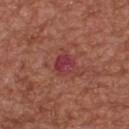patient — male, aged 63–67
site — the upper back
image-analysis metrics — a lesion color around L≈39 a*≈32 b*≈21 in CIELAB, roughly 8 lightness units darker than nearby skin, and a lesion-to-skin contrast of about 8.5 (normalized; higher = more distinct); border irregularity of about 2 on a 0–10 scale, a within-lesion color-variation index near 4/10, and peripheral color asymmetry of about 1.5
tile lighting — white-light illumination
size — ≈2.5 mm
image source — total-body-photography crop, ~15 mm field of view